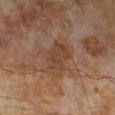Impression:
No biopsy was performed on this lesion — it was imaged during a full skin examination and was not determined to be concerning.
Clinical summary:
The subject is a male aged around 65. This image is a 15 mm lesion crop taken from a total-body photograph.